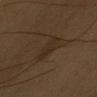workup = total-body-photography surveillance lesion; no biopsy | image = total-body-photography crop, ~15 mm field of view | patient = male, approximately 60 years of age | location = the right upper arm.